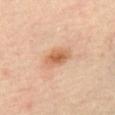| feature | finding |
|---|---|
| biopsy status | no biopsy performed (imaged during a skin exam) |
| subject | male, aged around 65 |
| image | total-body-photography crop, ~15 mm field of view |
| illumination | cross-polarized |
| location | the left thigh |
| lesion size | ~4 mm (longest diameter) |
| automated lesion analysis | an area of roughly 7.5 mm², an outline eccentricity of about 0.85 (0 = round, 1 = elongated), and a shape-asymmetry score of about 0.2 (0 = symmetric); a border-irregularity rating of about 2/10, a within-lesion color-variation index near 5/10, and radial color variation of about 1.5 |